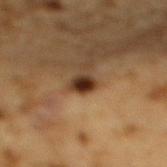biopsy status = imaged on a skin check; not biopsied
lesion size = about 2.5 mm
subject = male, roughly 85 years of age or older
image-analysis metrics = an average lesion color of about L≈28 a*≈17 b*≈26 (CIELAB) and about 15 CIELAB-L* units darker than the surrounding skin; a border-irregularity rating of about 2/10; an automated nevus-likeness rating near 95 out of 100
location = the mid back
illumination = cross-polarized illumination
imaging modality = ~15 mm crop, total-body skin-cancer survey